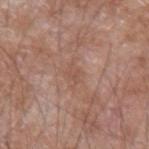| field | value |
|---|---|
| notes | catalogued during a skin exam; not biopsied |
| anatomic site | the left forearm |
| image source | ~15 mm crop, total-body skin-cancer survey |
| subject | male, in their 60s |